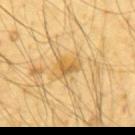Findings:
* workup: total-body-photography surveillance lesion; no biopsy
* patient: male, aged 63 to 67
* automated metrics: a lesion area of about 5 mm², a shape eccentricity near 0.65, and a shape-asymmetry score of about 0.5 (0 = symmetric); a border-irregularity rating of about 4.5/10, internal color variation of about 4 on a 0–10 scale, and peripheral color asymmetry of about 1.5; an automated nevus-likeness rating near 0 out of 100 and lesion-presence confidence of about 55/100
* illumination: cross-polarized
* anatomic site: the mid back
* image: total-body-photography crop, ~15 mm field of view
* lesion diameter: ≈3 mm